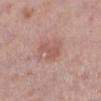Notes:
– workup · no biopsy performed (imaged during a skin exam)
– site · the right lower leg
– lighting · white-light
– image source · ~15 mm tile from a whole-body skin photo
– automated lesion analysis · a symmetry-axis asymmetry near 0.45; a mean CIELAB color near L≈56 a*≈23 b*≈24, a lesion–skin lightness drop of about 8, and a normalized border contrast of about 5.5; a border-irregularity index near 5/10 and a color-variation rating of about 1.5/10; a classifier nevus-likeness of about 15/100 and lesion-presence confidence of about 100/100
– subject · female, aged around 50